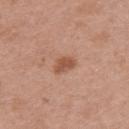workup: imaged on a skin check; not biopsied | location: the back | patient: female, in their 40s | lesion size: ≈2.5 mm | acquisition: 15 mm crop, total-body photography.